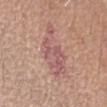Recorded during total-body skin imaging; not selected for excision or biopsy. Automated tile analysis of the lesion measured a lesion area of about 16 mm² and a shape eccentricity near 0.9. And it measured a lesion color around L≈57 a*≈23 b*≈22 in CIELAB, about 8 CIELAB-L* units darker than the surrounding skin, and a lesion-to-skin contrast of about 6.5 (normalized; higher = more distinct). It also reported a border-irregularity rating of about 5.5/10, internal color variation of about 4 on a 0–10 scale, and radial color variation of about 1.5. This image is a 15 mm lesion crop taken from a total-body photograph. The subject is a female aged 63 to 67. Captured under white-light illumination. Located on the left forearm.